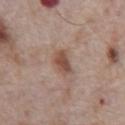follow-up: total-body-photography surveillance lesion; no biopsy
imaging modality: ~15 mm crop, total-body skin-cancer survey
size: ~3 mm (longest diameter)
patient: male, approximately 70 years of age
location: the abdomen
tile lighting: white-light
TBP lesion metrics: a footprint of about 6 mm², an eccentricity of roughly 0.7, and a symmetry-axis asymmetry near 0.25; roughly 11 lightness units darker than nearby skin and a lesion-to-skin contrast of about 8 (normalized; higher = more distinct); a border-irregularity index near 2/10 and a color-variation rating of about 2.5/10; an automated nevus-likeness rating near 85 out of 100 and a detector confidence of about 100 out of 100 that the crop contains a lesion